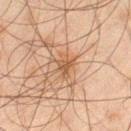Captured during whole-body skin photography for melanoma surveillance; the lesion was not biopsied.
Captured under cross-polarized illumination.
The lesion is located on the leg.
The subject is a male aged 43–47.
Automated image analysis of the tile measured a lesion area of about 5.5 mm² and two-axis asymmetry of about 0.35.
Approximately 3.5 mm at its widest.
A 15 mm close-up tile from a total-body photography series done for melanoma screening.